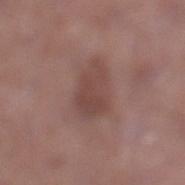Q: Was this lesion biopsied?
A: no biopsy performed (imaged during a skin exam)
Q: Automated lesion metrics?
A: a lesion area of about 11 mm², an outline eccentricity of about 0.7 (0 = round, 1 = elongated), and a shape-asymmetry score of about 0.25 (0 = symmetric); a mean CIELAB color near L≈45 a*≈20 b*≈22 and about 8 CIELAB-L* units darker than the surrounding skin; a nevus-likeness score of about 15/100 and lesion-presence confidence of about 100/100
Q: How was this image acquired?
A: 15 mm crop, total-body photography
Q: What are the patient's age and sex?
A: male, aged approximately 55
Q: Where on the body is the lesion?
A: the left lower leg
Q: What is the lesion's diameter?
A: ≈4.5 mm
Q: Illumination type?
A: white-light